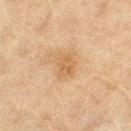The tile uses cross-polarized illumination. Automated image analysis of the tile measured a lesion color around L≈54 a*≈17 b*≈35 in CIELAB and a lesion–skin lightness drop of about 7. A lesion tile, about 15 mm wide, cut from a 3D total-body photograph. The subject is a female aged 78 to 82. The lesion is on the right thigh. Approximately 3 mm at its widest.